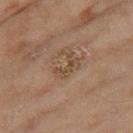Case summary:
• follow-up · catalogued during a skin exam; not biopsied
• patient · female, roughly 60 years of age
• imaging modality · ~15 mm crop, total-body skin-cancer survey
• lesion size · ≈3 mm
• anatomic site · the right thigh
• lighting · cross-polarized